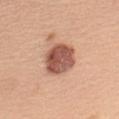This lesion was catalogued during total-body skin photography and was not selected for biopsy. The lesion is on the left upper arm. Longest diameter approximately 4 mm. The tile uses white-light illumination. A roughly 15 mm field-of-view crop from a total-body skin photograph. The patient is a female in their mid- to late 40s.